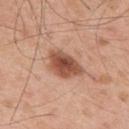Q: Is there a histopathology result?
A: total-body-photography surveillance lesion; no biopsy
Q: Patient demographics?
A: male, aged 53–57
Q: How was this image acquired?
A: ~15 mm tile from a whole-body skin photo
Q: What is the anatomic site?
A: the upper back